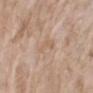  biopsy_status: not biopsied; imaged during a skin examination
  image:
    source: total-body photography crop
    field_of_view_mm: 15
  patient:
    sex: female
    age_approx: 75
  lighting: white-light
  site: left forearm
  automated_metrics:
    eccentricity: 0.9
    shape_asymmetry: 0.55
    cielab_L: 61
    cielab_a: 16
    cielab_b: 30
    vs_skin_contrast_norm: 4.5
    nevus_likeness_0_100: 0